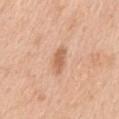follow-up = no biopsy performed (imaged during a skin exam); patient = male, aged around 50; image = total-body-photography crop, ~15 mm field of view; location = the mid back.